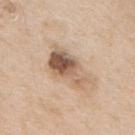Impression: No biopsy was performed on this lesion — it was imaged during a full skin examination and was not determined to be concerning. Clinical summary: The recorded lesion diameter is about 6 mm. The lesion is on the upper back. A male subject aged around 65. Cropped from a total-body skin-imaging series; the visible field is about 15 mm. Automated image analysis of the tile measured a shape-asymmetry score of about 0.35 (0 = symmetric). And it measured a lesion color around L≈58 a*≈17 b*≈30 in CIELAB and roughly 14 lightness units darker than nearby skin. The software also gave a color-variation rating of about 10/10 and radial color variation of about 4.5. The analysis additionally found a nevus-likeness score of about 70/100 and a detector confidence of about 100 out of 100 that the crop contains a lesion.